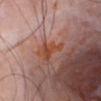{
  "biopsy_status": "not biopsied; imaged during a skin examination",
  "site": "abdomen",
  "patient": {
    "sex": "male",
    "age_approx": 65
  },
  "image": {
    "source": "total-body photography crop",
    "field_of_view_mm": 15
  }
}